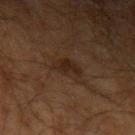Assessment: The lesion was photographed on a routine skin check and not biopsied; there is no pathology result. Image and clinical context: A male subject, in their 70s. A close-up tile cropped from a whole-body skin photograph, about 15 mm across. Captured under cross-polarized illumination. Measured at roughly 3 mm in maximum diameter. From the left upper arm.